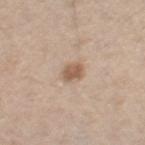Recorded during total-body skin imaging; not selected for excision or biopsy. Approximately 2.5 mm at its widest. This is a white-light tile. A 15 mm crop from a total-body photograph taken for skin-cancer surveillance. A male patient, approximately 70 years of age. An algorithmic analysis of the crop reported a lesion area of about 4 mm², an outline eccentricity of about 0.7 (0 = round, 1 = elongated), and a symmetry-axis asymmetry near 0.2. It also reported border irregularity of about 2 on a 0–10 scale, a within-lesion color-variation index near 1.5/10, and peripheral color asymmetry of about 0.5. The software also gave a classifier nevus-likeness of about 80/100. On the leg.